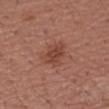Assessment: The lesion was photographed on a routine skin check and not biopsied; there is no pathology result. Image and clinical context: Longest diameter approximately 3.5 mm. A lesion tile, about 15 mm wide, cut from a 3D total-body photograph. A female subject, aged 53–57. This is a white-light tile. The lesion-visualizer software estimated a lesion color around L≈44 a*≈24 b*≈29 in CIELAB and roughly 8 lightness units darker than nearby skin. The software also gave a within-lesion color-variation index near 2/10 and peripheral color asymmetry of about 1. On the right upper arm.